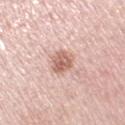<record>
<image>
  <source>total-body photography crop</source>
  <field_of_view_mm>15</field_of_view_mm>
</image>
<site>right upper arm</site>
<lesion_size>
  <long_diameter_mm_approx>3.0</long_diameter_mm_approx>
</lesion_size>
<lighting>white-light</lighting>
<automated_metrics>
  <area_mm2_approx>6.5</area_mm2_approx>
  <eccentricity>0.6</eccentricity>
  <cielab_L>63</cielab_L>
  <cielab_a>22</cielab_a>
  <cielab_b>26</cielab_b>
  <vs_skin_darker_L>13.0</vs_skin_darker_L>
  <vs_skin_contrast_norm>8.0</vs_skin_contrast_norm>
  <border_irregularity_0_10>1.5</border_irregularity_0_10>
  <color_variation_0_10>4.5</color_variation_0_10>
  <peripheral_color_asymmetry>1.5</peripheral_color_asymmetry>
  <nevus_likeness_0_100>85</nevus_likeness_0_100>
</automated_metrics>
<patient>
  <sex>female</sex>
  <age_approx>65</age_approx>
</patient>
</record>